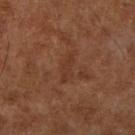Assessment: Captured during whole-body skin photography for melanoma surveillance; the lesion was not biopsied. Context: The lesion is on the right lower leg. Approximately 3.5 mm at its widest. A 15 mm close-up tile from a total-body photography series done for melanoma screening. A patient in their mid-60s. Automated tile analysis of the lesion measured border irregularity of about 4 on a 0–10 scale, a within-lesion color-variation index near 1.5/10, and peripheral color asymmetry of about 0.5. It also reported a classifier nevus-likeness of about 0/100. This is a cross-polarized tile.